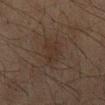notes = catalogued during a skin exam; not biopsied | patient = male, about 45 years old | acquisition = 15 mm crop, total-body photography | automated lesion analysis = an eccentricity of roughly 0.9 and two-axis asymmetry of about 0.35; a mean CIELAB color near L≈24 a*≈11 b*≈19, roughly 4 lightness units darker than nearby skin, and a normalized lesion–skin contrast near 5; a classifier nevus-likeness of about 0/100 and a detector confidence of about 100 out of 100 that the crop contains a lesion | tile lighting = cross-polarized | body site = the mid back | lesion diameter = about 5 mm.